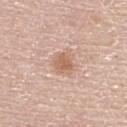Assessment: Part of a total-body skin-imaging series; this lesion was reviewed on a skin check and was not flagged for biopsy. Background: Located on the upper back. A male patient, about 60 years old. A 15 mm close-up tile from a total-body photography series done for melanoma screening.